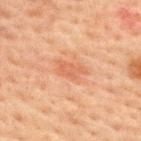Findings:
- biopsy status: no biopsy performed (imaged during a skin exam)
- TBP lesion metrics: about 7 CIELAB-L* units darker than the surrounding skin and a normalized lesion–skin contrast near 5; border irregularity of about 3.5 on a 0–10 scale, internal color variation of about 1.5 on a 0–10 scale, and peripheral color asymmetry of about 0.5
- patient: male, in their 60s
- imaging modality: total-body-photography crop, ~15 mm field of view
- tile lighting: cross-polarized illumination
- diameter: ≈3.5 mm
- site: the upper back